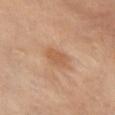Q: Was a biopsy performed?
A: total-body-photography surveillance lesion; no biopsy
Q: What is the imaging modality?
A: ~15 mm crop, total-body skin-cancer survey
Q: Lesion size?
A: ≈3 mm
Q: Lesion location?
A: the chest
Q: Who is the patient?
A: female, approximately 55 years of age
Q: What did automated image analysis measure?
A: a lesion area of about 4.5 mm² and a shape eccentricity near 0.8; a border-irregularity rating of about 2.5/10, a within-lesion color-variation index near 1.5/10, and radial color variation of about 0.5; an automated nevus-likeness rating near 10 out of 100 and a lesion-detection confidence of about 100/100